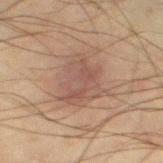Part of a total-body skin-imaging series; this lesion was reviewed on a skin check and was not flagged for biopsy.